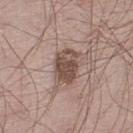The lesion was photographed on a routine skin check and not biopsied; there is no pathology result. A male patient, aged 63 to 67. The lesion is located on the left lower leg. A 15 mm close-up tile from a total-body photography series done for melanoma screening. Imaged with white-light lighting.